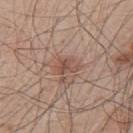Recorded during total-body skin imaging; not selected for excision or biopsy. Measured at roughly 3 mm in maximum diameter. This is a white-light tile. A region of skin cropped from a whole-body photographic capture, roughly 15 mm wide. On the back. A male subject aged 48 to 52.